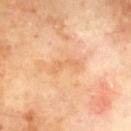biopsy status: catalogued during a skin exam; not biopsied
image: 15 mm crop, total-body photography
body site: the upper back
subject: male, aged 68–72